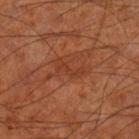automated lesion analysis: a lesion area of about 3.5 mm², a shape eccentricity near 0.9, and two-axis asymmetry of about 0.5; a border-irregularity index near 7/10, a color-variation rating of about 0/10, and a peripheral color-asymmetry measure near 0; a nevus-likeness score of about 0/100 and lesion-presence confidence of about 95/100
body site: the leg
patient: male, aged approximately 70
lesion diameter: about 3.5 mm
lighting: cross-polarized illumination
acquisition: 15 mm crop, total-body photography